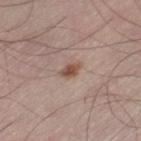Q: Is there a histopathology result?
A: imaged on a skin check; not biopsied
Q: Illumination type?
A: white-light illumination
Q: Patient demographics?
A: male, in their mid-50s
Q: Where on the body is the lesion?
A: the left thigh
Q: How large is the lesion?
A: ~2.5 mm (longest diameter)
Q: What kind of image is this?
A: 15 mm crop, total-body photography